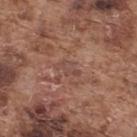No biopsy was performed on this lesion — it was imaged during a full skin examination and was not determined to be concerning.
A male patient, in their mid-70s.
The lesion's longest dimension is about 3 mm.
Captured under white-light illumination.
The lesion is located on the back.
A roughly 15 mm field-of-view crop from a total-body skin photograph.